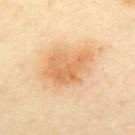This lesion was catalogued during total-body skin photography and was not selected for biopsy.
Imaged with cross-polarized lighting.
Cropped from a total-body skin-imaging series; the visible field is about 15 mm.
A female subject, aged around 40.
From the upper back.